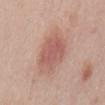Imaged during a routine full-body skin examination; the lesion was not biopsied and no histopathology is available.